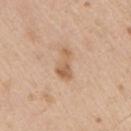No biopsy was performed on this lesion — it was imaged during a full skin examination and was not determined to be concerning. Imaged with white-light lighting. A 15 mm crop from a total-body photograph taken for skin-cancer surveillance. The lesion is on the right upper arm. A male subject about 65 years old. Longest diameter approximately 3.5 mm.